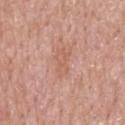No biopsy was performed on this lesion — it was imaged during a full skin examination and was not determined to be concerning.
The patient is a male approximately 55 years of age.
From the back.
A lesion tile, about 15 mm wide, cut from a 3D total-body photograph.
About 3.5 mm across.